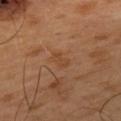This lesion was catalogued during total-body skin photography and was not selected for biopsy.
The lesion is on the upper back.
The subject is a male about 50 years old.
A 15 mm close-up tile from a total-body photography series done for melanoma screening.